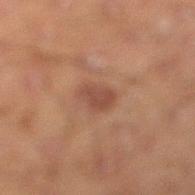| field | value |
|---|---|
| notes | total-body-photography surveillance lesion; no biopsy |
| lesion size | about 3 mm |
| image | 15 mm crop, total-body photography |
| site | the right lower leg |
| patient | male, roughly 45 years of age |